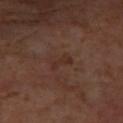| feature | finding |
|---|---|
| tile lighting | cross-polarized |
| imaging modality | 15 mm crop, total-body photography |
| body site | the left forearm |
| lesion diameter | ~3 mm (longest diameter) |
| subject | female, approximately 50 years of age |